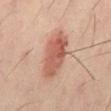{
  "biopsy_status": "not biopsied; imaged during a skin examination",
  "patient": {
    "sex": "male",
    "age_approx": 40
  },
  "lighting": "cross-polarized",
  "image": {
    "source": "total-body photography crop",
    "field_of_view_mm": 15
  },
  "lesion_size": {
    "long_diameter_mm_approx": 6.0
  },
  "site": "mid back"
}